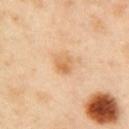Assessment: Imaged during a routine full-body skin examination; the lesion was not biopsied and no histopathology is available. Clinical summary: The lesion is on the arm. A 15 mm crop from a total-body photograph taken for skin-cancer surveillance. Measured at roughly 2.5 mm in maximum diameter. This is a cross-polarized tile. The patient is a female aged 38 to 42. The total-body-photography lesion software estimated a within-lesion color-variation index near 4/10 and a peripheral color-asymmetry measure near 1.5.